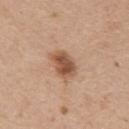image-analysis metrics = an area of roughly 8 mm² and an eccentricity of roughly 0.75; an average lesion color of about L≈53 a*≈21 b*≈32 (CIELAB), roughly 13 lightness units darker than nearby skin, and a normalized border contrast of about 9; border irregularity of about 1.5 on a 0–10 scale, a within-lesion color-variation index near 4/10, and a peripheral color-asymmetry measure near 1.5; a classifier nevus-likeness of about 90/100 and lesion-presence confidence of about 100/100 | image = total-body-photography crop, ~15 mm field of view | tile lighting = white-light | site = the arm | patient = female, about 45 years old.